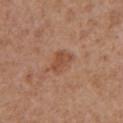Assessment: Part of a total-body skin-imaging series; this lesion was reviewed on a skin check and was not flagged for biopsy. Acquisition and patient details: The subject is a male aged around 65. The tile uses white-light illumination. On the chest. A 15 mm crop from a total-body photograph taken for skin-cancer surveillance. Longest diameter approximately 3 mm.